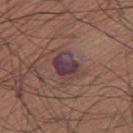Imaged during a routine full-body skin examination; the lesion was not biopsied and no histopathology is available.
Captured under white-light illumination.
Located on the leg.
A region of skin cropped from a whole-body photographic capture, roughly 15 mm wide.
Approximately 3.5 mm at its widest.
The patient is a male aged approximately 60.